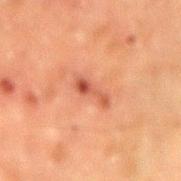Case summary:
• notes — total-body-photography surveillance lesion; no biopsy
• imaging modality — total-body-photography crop, ~15 mm field of view
• subject — male, aged around 60
• site — the arm